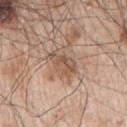{"biopsy_status": "not biopsied; imaged during a skin examination", "patient": {"sex": "male", "age_approx": 65}, "site": "right upper arm", "lesion_size": {"long_diameter_mm_approx": 4.0}, "lighting": "white-light", "image": {"source": "total-body photography crop", "field_of_view_mm": 15}}